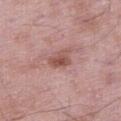Imaged during a routine full-body skin examination; the lesion was not biopsied and no histopathology is available. Measured at roughly 2.5 mm in maximum diameter. Located on the right thigh. The subject is a male aged approximately 50. The tile uses white-light illumination. A lesion tile, about 15 mm wide, cut from a 3D total-body photograph. Automated tile analysis of the lesion measured a lesion color around L≈51 a*≈23 b*≈25 in CIELAB and a lesion–skin lightness drop of about 11. The analysis additionally found a color-variation rating of about 2.5/10 and peripheral color asymmetry of about 0.5. The analysis additionally found an automated nevus-likeness rating near 30 out of 100.